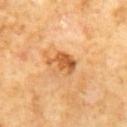Recorded during total-body skin imaging; not selected for excision or biopsy.
A male patient aged 58 to 62.
Longest diameter approximately 3.5 mm.
The lesion is located on the abdomen.
Cropped from a whole-body photographic skin survey; the tile spans about 15 mm.
The total-body-photography lesion software estimated a lesion color around L≈56 a*≈25 b*≈43 in CIELAB, about 12 CIELAB-L* units darker than the surrounding skin, and a normalized border contrast of about 8. It also reported a detector confidence of about 100 out of 100 that the crop contains a lesion.